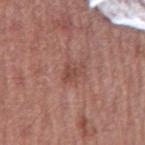Clinical impression:
Captured during whole-body skin photography for melanoma surveillance; the lesion was not biopsied.
Background:
From the left upper arm. A 15 mm crop from a total-body photograph taken for skin-cancer surveillance. The subject is a male aged 43 to 47. Captured under white-light illumination. The total-body-photography lesion software estimated a lesion color around L≈47 a*≈23 b*≈26 in CIELAB and a lesion–skin lightness drop of about 8. It also reported an automated nevus-likeness rating near 0 out of 100 and a detector confidence of about 100 out of 100 that the crop contains a lesion. About 3 mm across.